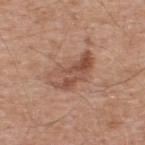The lesion was photographed on a routine skin check and not biopsied; there is no pathology result. The patient is a male aged 53 to 57. Longest diameter approximately 5.5 mm. From the back. The total-body-photography lesion software estimated a footprint of about 13 mm², an outline eccentricity of about 0.85 (0 = round, 1 = elongated), and a symmetry-axis asymmetry near 0.35. It also reported internal color variation of about 8 on a 0–10 scale and radial color variation of about 3. And it measured a lesion-detection confidence of about 100/100. Imaged with white-light lighting. A roughly 15 mm field-of-view crop from a total-body skin photograph.